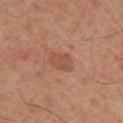Impression: The lesion was tiled from a total-body skin photograph and was not biopsied. Image and clinical context: A close-up tile cropped from a whole-body skin photograph, about 15 mm across. The recorded lesion diameter is about 3 mm. Imaged with cross-polarized lighting. Located on the upper back. A male patient aged around 55. Automated tile analysis of the lesion measured a mean CIELAB color near L≈51 a*≈22 b*≈33, about 7 CIELAB-L* units darker than the surrounding skin, and a normalized border contrast of about 5.5. And it measured an automated nevus-likeness rating near 5 out of 100.